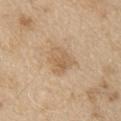Q: Is there a histopathology result?
A: catalogued during a skin exam; not biopsied
Q: Who is the patient?
A: male, aged around 70
Q: What kind of image is this?
A: ~15 mm crop, total-body skin-cancer survey
Q: Lesion location?
A: the left upper arm